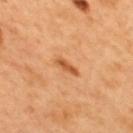Part of a total-body skin-imaging series; this lesion was reviewed on a skin check and was not flagged for biopsy.
A male subject, approximately 50 years of age.
From the back.
This is a cross-polarized tile.
A 15 mm close-up extracted from a 3D total-body photography capture.
The lesion's longest dimension is about 3.5 mm.